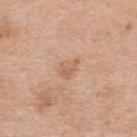Image and clinical context: A female patient, in their 40s. From the upper back. The lesion's longest dimension is about 3 mm. This image is a 15 mm lesion crop taken from a total-body photograph. The total-body-photography lesion software estimated a lesion area of about 4 mm² and an eccentricity of roughly 0.7. And it measured a lesion-detection confidence of about 100/100.